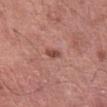Recorded during total-body skin imaging; not selected for excision or biopsy. Approximately 2.5 mm at its widest. A lesion tile, about 15 mm wide, cut from a 3D total-body photograph. A female subject, aged 58–62. The lesion is on the abdomen. The lesion-visualizer software estimated an area of roughly 3 mm², an outline eccentricity of about 0.9 (0 = round, 1 = elongated), and a symmetry-axis asymmetry near 0.2. The software also gave a lesion color around L≈49 a*≈25 b*≈27 in CIELAB and about 11 CIELAB-L* units darker than the surrounding skin. Imaged with white-light lighting.